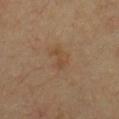biopsy status = total-body-photography surveillance lesion; no biopsy
subject = roughly 60 years of age
lighting = cross-polarized illumination
body site = the chest
image = 15 mm crop, total-body photography
lesion diameter = ~2.5 mm (longest diameter)
image-analysis metrics = a symmetry-axis asymmetry near 0.35; a lesion color around L≈45 a*≈17 b*≈31 in CIELAB, roughly 6 lightness units darker than nearby skin, and a normalized border contrast of about 5; a border-irregularity rating of about 4.5/10, a within-lesion color-variation index near 0/10, and radial color variation of about 0